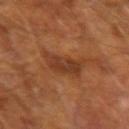workup=imaged on a skin check; not biopsied | patient=male, roughly 65 years of age | site=the left forearm | illumination=cross-polarized | lesion size=≈4.5 mm | acquisition=~15 mm crop, total-body skin-cancer survey.